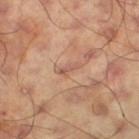Q: How was the tile lit?
A: cross-polarized
Q: Patient demographics?
A: male, aged around 60
Q: How was this image acquired?
A: ~15 mm tile from a whole-body skin photo
Q: Lesion location?
A: the left thigh
Q: Lesion size?
A: ≈3 mm